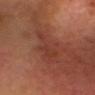Q: Is there a histopathology result?
A: no biopsy performed (imaged during a skin exam)
Q: How was this image acquired?
A: ~15 mm crop, total-body skin-cancer survey
Q: What is the lesion's diameter?
A: ≈4.5 mm
Q: What are the patient's age and sex?
A: male, about 65 years old
Q: Illumination type?
A: cross-polarized